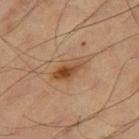Q: Is there a histopathology result?
A: catalogued during a skin exam; not biopsied
Q: Lesion location?
A: the right thigh
Q: What are the patient's age and sex?
A: male, aged approximately 60
Q: What is the imaging modality?
A: ~15 mm crop, total-body skin-cancer survey
Q: How was the tile lit?
A: cross-polarized illumination
Q: Automated lesion metrics?
A: a lesion color around L≈49 a*≈20 b*≈34 in CIELAB, roughly 10 lightness units darker than nearby skin, and a normalized lesion–skin contrast near 8; a nevus-likeness score of about 95/100